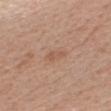Case summary:
- notes — no biopsy performed (imaged during a skin exam)
- lighting — white-light
- body site — the head or neck
- subject — female, roughly 50 years of age
- size — ≈2.5 mm
- imaging modality — total-body-photography crop, ~15 mm field of view